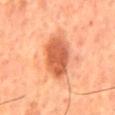Part of a total-body skin-imaging series; this lesion was reviewed on a skin check and was not flagged for biopsy.